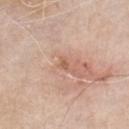  biopsy_status: not biopsied; imaged during a skin examination
  image:
    source: total-body photography crop
    field_of_view_mm: 15
  lighting: white-light
  lesion_size:
    long_diameter_mm_approx: 1.0
  patient:
    sex: male
    age_approx: 80
  site: front of the torso